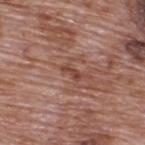From the upper back. A 15 mm close-up tile from a total-body photography series done for melanoma screening. The patient is a male in their 70s.